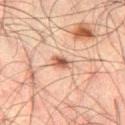The lesion was photographed on a routine skin check and not biopsied; there is no pathology result. Automated image analysis of the tile measured a lesion area of about 4 mm², an eccentricity of roughly 0.75, and two-axis asymmetry of about 0.2. And it measured a border-irregularity index near 2/10 and internal color variation of about 4.5 on a 0–10 scale. On the left thigh. A male subject aged around 50. Cropped from a total-body skin-imaging series; the visible field is about 15 mm. The recorded lesion diameter is about 2.5 mm.